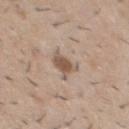Recorded during total-body skin imaging; not selected for excision or biopsy.
Located on the chest.
A male patient roughly 30 years of age.
Imaged with white-light lighting.
The total-body-photography lesion software estimated a lesion color around L≈54 a*≈16 b*≈26 in CIELAB and a lesion-to-skin contrast of about 8 (normalized; higher = more distinct). It also reported internal color variation of about 3.5 on a 0–10 scale and peripheral color asymmetry of about 1. The software also gave a classifier nevus-likeness of about 90/100 and a lesion-detection confidence of about 100/100.
A close-up tile cropped from a whole-body skin photograph, about 15 mm across.